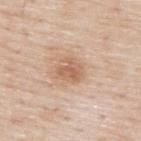No biopsy was performed on this lesion — it was imaged during a full skin examination and was not determined to be concerning.
The total-body-photography lesion software estimated a lesion area of about 6.5 mm², a shape eccentricity near 0.5, and a symmetry-axis asymmetry near 0.25. It also reported an average lesion color of about L≈62 a*≈20 b*≈32 (CIELAB), about 9 CIELAB-L* units darker than the surrounding skin, and a normalized border contrast of about 6.5. The analysis additionally found a detector confidence of about 100 out of 100 that the crop contains a lesion.
From the upper back.
This is a white-light tile.
Cropped from a total-body skin-imaging series; the visible field is about 15 mm.
Longest diameter approximately 3 mm.
The subject is a male about 80 years old.